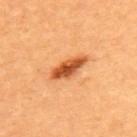Clinical impression: Captured during whole-body skin photography for melanoma surveillance; the lesion was not biopsied. Background: A 15 mm close-up tile from a total-body photography series done for melanoma screening. The lesion is on the upper back. The subject is a female in their mid- to late 30s. The tile uses cross-polarized illumination. Automated image analysis of the tile measured border irregularity of about 2.5 on a 0–10 scale, internal color variation of about 4 on a 0–10 scale, and radial color variation of about 1.